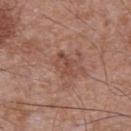location = the abdomen; acquisition = ~15 mm tile from a whole-body skin photo; lesion size = ≈3.5 mm; subject = male, roughly 70 years of age; tile lighting = white-light illumination.